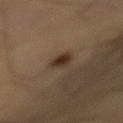Assessment: No biopsy was performed on this lesion — it was imaged during a full skin examination and was not determined to be concerning. Context: The lesion-visualizer software estimated an outline eccentricity of about 0.9 (0 = round, 1 = elongated) and two-axis asymmetry of about 0.25. Located on the mid back. A roughly 15 mm field-of-view crop from a total-body skin photograph. The subject is a male approximately 55 years of age. Approximately 4 mm at its widest. Imaged with cross-polarized lighting.